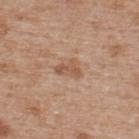Q: Is there a histopathology result?
A: no biopsy performed (imaged during a skin exam)
Q: What is the anatomic site?
A: the upper back
Q: What lighting was used for the tile?
A: white-light
Q: How was this image acquired?
A: ~15 mm tile from a whole-body skin photo
Q: What is the lesion's diameter?
A: ~3 mm (longest diameter)
Q: What are the patient's age and sex?
A: male, about 65 years old